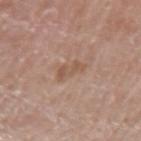Imaged during a routine full-body skin examination; the lesion was not biopsied and no histopathology is available. Automated tile analysis of the lesion measured an area of roughly 3.5 mm² and an outline eccentricity of about 0.85 (0 = round, 1 = elongated). It also reported an average lesion color of about L≈54 a*≈19 b*≈29 (CIELAB). The analysis additionally found a border-irregularity rating of about 4/10, a within-lesion color-variation index near 2/10, and peripheral color asymmetry of about 0.5. The analysis additionally found an automated nevus-likeness rating near 0 out of 100 and a detector confidence of about 100 out of 100 that the crop contains a lesion. The recorded lesion diameter is about 3 mm. The tile uses white-light illumination. From the left upper arm. This image is a 15 mm lesion crop taken from a total-body photograph. The subject is a male aged 68–72.